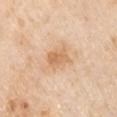Assessment:
Imaged during a routine full-body skin examination; the lesion was not biopsied and no histopathology is available.
Acquisition and patient details:
A male subject in their mid- to late 70s. Measured at roughly 3 mm in maximum diameter. The lesion is on the left upper arm. Captured under white-light illumination. A region of skin cropped from a whole-body photographic capture, roughly 15 mm wide.